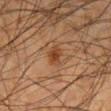- follow-up: imaged on a skin check; not biopsied
- patient: male, approximately 35 years of age
- lesion size: ~2 mm (longest diameter)
- lighting: cross-polarized
- site: the left lower leg
- image: ~15 mm tile from a whole-body skin photo
- automated metrics: a footprint of about 4 mm², an outline eccentricity of about 0.3 (0 = round, 1 = elongated), and a symmetry-axis asymmetry near 0.2; border irregularity of about 1.5 on a 0–10 scale and a color-variation rating of about 3/10